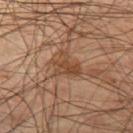The lesion was tiled from a total-body skin photograph and was not biopsied. The recorded lesion diameter is about 3.5 mm. Imaged with cross-polarized lighting. The patient is a male about 55 years old. From the left thigh. This image is a 15 mm lesion crop taken from a total-body photograph.